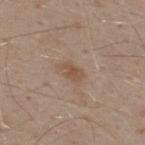Impression:
The lesion was photographed on a routine skin check and not biopsied; there is no pathology result.
Context:
About 2.5 mm across. From the upper back. The subject is a male in their 30s. Cropped from a total-body skin-imaging series; the visible field is about 15 mm.